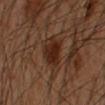Clinical impression: Part of a total-body skin-imaging series; this lesion was reviewed on a skin check and was not flagged for biopsy. Image and clinical context: Cropped from a whole-body photographic skin survey; the tile spans about 15 mm. The lesion is located on the left forearm. A male subject, aged approximately 50.